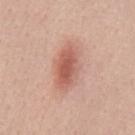| field | value |
|---|---|
| workup | no biopsy performed (imaged during a skin exam) |
| lesion size | ~5.5 mm (longest diameter) |
| acquisition | ~15 mm crop, total-body skin-cancer survey |
| anatomic site | the back |
| automated lesion analysis | a lesion–skin lightness drop of about 12 and a normalized border contrast of about 7.5 |
| patient | male, aged 53 to 57 |